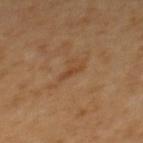| field | value |
|---|---|
| biopsy status | total-body-photography surveillance lesion; no biopsy |
| anatomic site | the upper back |
| patient | female, about 65 years old |
| lesion size | ~2.5 mm (longest diameter) |
| imaging modality | total-body-photography crop, ~15 mm field of view |
| lighting | cross-polarized illumination |
| automated lesion analysis | an outline eccentricity of about 0.95 (0 = round, 1 = elongated); internal color variation of about 0 on a 0–10 scale |